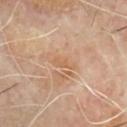<record>
<biopsy_status>not biopsied; imaged during a skin examination</biopsy_status>
</record>